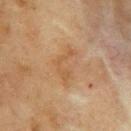tile lighting=cross-polarized
patient=female, about 70 years old
anatomic site=the right upper arm
lesion diameter=about 4 mm
acquisition=total-body-photography crop, ~15 mm field of view
automated lesion analysis=about 6 CIELAB-L* units darker than the surrounding skin; a border-irregularity index near 9.5/10, internal color variation of about 0.5 on a 0–10 scale, and radial color variation of about 0